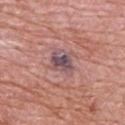Part of a total-body skin-imaging series; this lesion was reviewed on a skin check and was not flagged for biopsy.
On the back.
The patient is a male in their 60s.
A 15 mm close-up extracted from a 3D total-body photography capture.